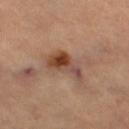Recorded during total-body skin imaging; not selected for excision or biopsy.